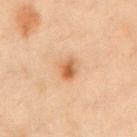Part of a total-body skin-imaging series; this lesion was reviewed on a skin check and was not flagged for biopsy.
A male subject, roughly 55 years of age.
An algorithmic analysis of the crop reported a lesion-detection confidence of about 100/100.
The lesion is on the chest.
The lesion's longest dimension is about 2.5 mm.
The tile uses cross-polarized illumination.
A 15 mm close-up tile from a total-body photography series done for melanoma screening.